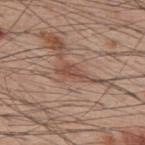Assessment:
Captured during whole-body skin photography for melanoma surveillance; the lesion was not biopsied.
Context:
A male patient, about 60 years old. About 4.5 mm across. A region of skin cropped from a whole-body photographic capture, roughly 15 mm wide. The tile uses white-light illumination. On the upper back.